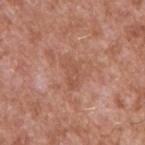biopsy_status: not biopsied; imaged during a skin examination
lighting: white-light
automated_metrics:
  vs_skin_darker_L: 6.0
image:
  source: total-body photography crop
  field_of_view_mm: 15
site: right upper arm
patient:
  sex: male
  age_approx: 45
lesion_size:
  long_diameter_mm_approx: 3.5